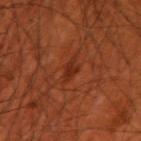biopsy status: catalogued during a skin exam; not biopsied | anatomic site: the right upper arm | illumination: cross-polarized | image-analysis metrics: a lesion area of about 3 mm², a shape eccentricity near 0.85, and two-axis asymmetry of about 0.3; a within-lesion color-variation index near 1/10 and a peripheral color-asymmetry measure near 0.5; a classifier nevus-likeness of about 0/100 and lesion-presence confidence of about 95/100 | patient: male, roughly 70 years of age | lesion diameter: ≈2.5 mm | image source: 15 mm crop, total-body photography.